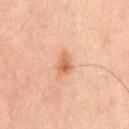This lesion was catalogued during total-body skin photography and was not selected for biopsy.
Captured under cross-polarized illumination.
A lesion tile, about 15 mm wide, cut from a 3D total-body photograph.
The total-body-photography lesion software estimated a footprint of about 4 mm², an eccentricity of roughly 0.8, and two-axis asymmetry of about 0.25. The software also gave a lesion color around L≈60 a*≈25 b*≈35 in CIELAB, about 10 CIELAB-L* units darker than the surrounding skin, and a lesion-to-skin contrast of about 7.5 (normalized; higher = more distinct). The analysis additionally found a border-irregularity rating of about 2.5/10 and peripheral color asymmetry of about 1.5. And it measured lesion-presence confidence of about 100/100.
A male patient aged 43 to 47.
The lesion is located on the chest.